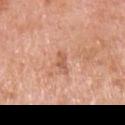Recorded during total-body skin imaging; not selected for excision or biopsy. Approximately 3 mm at its widest. The lesion is on the chest. The subject is a male aged 68 to 72. This is a white-light tile. A 15 mm close-up extracted from a 3D total-body photography capture. An algorithmic analysis of the crop reported a footprint of about 3.5 mm² and a shape-asymmetry score of about 0.4 (0 = symmetric). The analysis additionally found a mean CIELAB color near L≈59 a*≈25 b*≈33 and roughly 9 lightness units darker than nearby skin. The analysis additionally found border irregularity of about 4.5 on a 0–10 scale, internal color variation of about 1 on a 0–10 scale, and radial color variation of about 0. And it measured a nevus-likeness score of about 0/100 and a lesion-detection confidence of about 100/100.